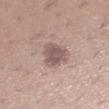workup = no biopsy performed (imaged during a skin exam) | lesion diameter = ≈3.5 mm | illumination = white-light | subject = female, aged approximately 30 | acquisition = ~15 mm tile from a whole-body skin photo | location = the left lower leg | TBP lesion metrics = a border-irregularity rating of about 3/10, a within-lesion color-variation index near 3.5/10, and peripheral color asymmetry of about 1.